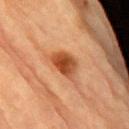Part of a total-body skin-imaging series; this lesion was reviewed on a skin check and was not flagged for biopsy. A male subject, about 85 years old or older. The lesion's longest dimension is about 4 mm. A roughly 15 mm field-of-view crop from a total-body skin photograph. The lesion is on the right upper arm. Automated image analysis of the tile measured two-axis asymmetry of about 0.3. The analysis additionally found a lesion color around L≈44 a*≈25 b*≈36 in CIELAB, a lesion–skin lightness drop of about 12, and a normalized lesion–skin contrast near 9.5. The software also gave a detector confidence of about 100 out of 100 that the crop contains a lesion.